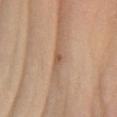Impression: Part of a total-body skin-imaging series; this lesion was reviewed on a skin check and was not flagged for biopsy. Background: Measured at roughly 3 mm in maximum diameter. A male patient aged 58–62. Imaged with white-light lighting. The total-body-photography lesion software estimated a footprint of about 3.5 mm², an outline eccentricity of about 0.9 (0 = round, 1 = elongated), and a shape-asymmetry score of about 0.35 (0 = symmetric). The analysis additionally found a lesion color around L≈56 a*≈18 b*≈31 in CIELAB, roughly 9 lightness units darker than nearby skin, and a normalized border contrast of about 6. And it measured a border-irregularity rating of about 4/10, a color-variation rating of about 2/10, and peripheral color asymmetry of about 0.5. On the right forearm. A 15 mm close-up extracted from a 3D total-body photography capture.